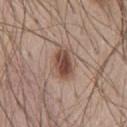Notes:
• follow-up: catalogued during a skin exam; not biopsied
• size: ≈4 mm
• location: the chest
• imaging modality: 15 mm crop, total-body photography
• patient: male, about 45 years old